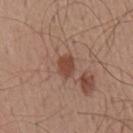This lesion was catalogued during total-body skin photography and was not selected for biopsy. A 15 mm close-up extracted from a 3D total-body photography capture. The subject is a male aged approximately 55. Measured at roughly 2.5 mm in maximum diameter. From the chest. An algorithmic analysis of the crop reported a mean CIELAB color near L≈45 a*≈22 b*≈28, about 10 CIELAB-L* units darker than the surrounding skin, and a normalized lesion–skin contrast near 8. Captured under white-light illumination.